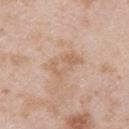Impression:
Part of a total-body skin-imaging series; this lesion was reviewed on a skin check and was not flagged for biopsy.
Clinical summary:
A male subject aged approximately 25. The lesion is on the upper back. Cropped from a whole-body photographic skin survey; the tile spans about 15 mm. Approximately 4 mm at its widest.